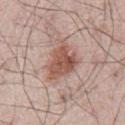Q: Is there a histopathology result?
A: catalogued during a skin exam; not biopsied
Q: What kind of image is this?
A: total-body-photography crop, ~15 mm field of view
Q: How large is the lesion?
A: ≈5 mm
Q: Who is the patient?
A: male, aged approximately 65
Q: What lighting was used for the tile?
A: white-light illumination
Q: Where on the body is the lesion?
A: the abdomen